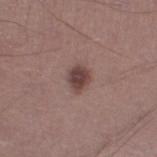Q: Is there a histopathology result?
A: no biopsy performed (imaged during a skin exam)
Q: What are the patient's age and sex?
A: male, aged 43 to 47
Q: Lesion location?
A: the leg
Q: What is the imaging modality?
A: ~15 mm crop, total-body skin-cancer survey